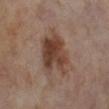  biopsy_status: not biopsied; imaged during a skin examination
  image:
    source: total-body photography crop
    field_of_view_mm: 15
  automated_metrics:
    area_mm2_approx: 17.0
    eccentricity: 0.6
    shape_asymmetry: 0.25
    cielab_L: 42
    cielab_a: 18
    cielab_b: 26
    vs_skin_darker_L: 11.0
    vs_skin_contrast_norm: 9.5
    color_variation_0_10: 5.5
    peripheral_color_asymmetry: 2.0
  patient:
    sex: female
    age_approx: 55
  lighting: cross-polarized
  site: leg